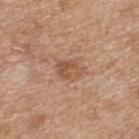| field | value |
|---|---|
| follow-up | total-body-photography surveillance lesion; no biopsy |
| image | 15 mm crop, total-body photography |
| subject | male, aged around 70 |
| site | the upper back |
| tile lighting | white-light illumination |
| image-analysis metrics | a lesion area of about 5.5 mm²; a normalized lesion–skin contrast near 6; lesion-presence confidence of about 100/100 |
| diameter | ~3 mm (longest diameter) |